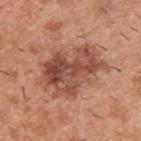Findings:
• workup — catalogued during a skin exam; not biopsied
• illumination — white-light
• anatomic site — the upper back
• automated metrics — an area of roughly 30 mm², an eccentricity of roughly 0.75, and a shape-asymmetry score of about 0.25 (0 = symmetric)
• imaging modality — ~15 mm tile from a whole-body skin photo
• patient — male, aged 38 to 42